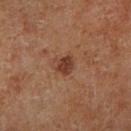Captured during whole-body skin photography for melanoma surveillance; the lesion was not biopsied. A 15 mm crop from a total-body photograph taken for skin-cancer surveillance. A male subject, roughly 70 years of age. The lesion is located on the right lower leg. About 2.5 mm across.